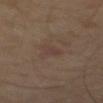{
  "biopsy_status": "not biopsied; imaged during a skin examination",
  "image": {
    "source": "total-body photography crop",
    "field_of_view_mm": 15
  },
  "patient": {
    "sex": "male",
    "age_approx": 55
  },
  "site": "back"
}